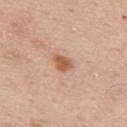Clinical impression:
No biopsy was performed on this lesion — it was imaged during a full skin examination and was not determined to be concerning.
Acquisition and patient details:
A 15 mm close-up tile from a total-body photography series done for melanoma screening. On the front of the torso. A male patient, aged 58–62. The tile uses white-light illumination. The lesion's longest dimension is about 2.5 mm.